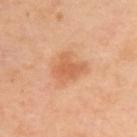| field | value |
|---|---|
| illumination | cross-polarized illumination |
| imaging modality | 15 mm crop, total-body photography |
| subject | female, roughly 40 years of age |
| anatomic site | the back |